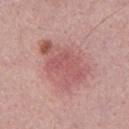follow-up: no biopsy performed (imaged during a skin exam) | acquisition: 15 mm crop, total-body photography | diameter: about 6.5 mm | patient: male, approximately 30 years of age | automated lesion analysis: a mean CIELAB color near L≈56 a*≈26 b*≈23; border irregularity of about 5.5 on a 0–10 scale | lighting: white-light.